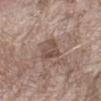This lesion was catalogued during total-body skin photography and was not selected for biopsy. A male patient about 70 years old. The tile uses white-light illumination. Located on the arm. The recorded lesion diameter is about 4 mm. A close-up tile cropped from a whole-body skin photograph, about 15 mm across. The lesion-visualizer software estimated an area of roughly 6.5 mm² and a symmetry-axis asymmetry near 0.4. And it measured a lesion color around L≈49 a*≈16 b*≈24 in CIELAB, a lesion–skin lightness drop of about 9, and a normalized border contrast of about 6.5. It also reported lesion-presence confidence of about 100/100.